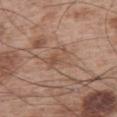• notes · total-body-photography surveillance lesion; no biopsy
• acquisition · ~15 mm crop, total-body skin-cancer survey
• lighting · white-light illumination
• patient · male, roughly 65 years of age
• automated metrics · an area of roughly 7 mm², an eccentricity of roughly 0.6, and a symmetry-axis asymmetry near 0.4; a lesion–skin lightness drop of about 7 and a lesion-to-skin contrast of about 5 (normalized; higher = more distinct)
• lesion diameter · ~3.5 mm (longest diameter)
• body site · the left upper arm